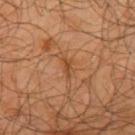<record>
  <biopsy_status>not biopsied; imaged during a skin examination</biopsy_status>
  <image>
    <source>total-body photography crop</source>
    <field_of_view_mm>15</field_of_view_mm>
  </image>
  <automated_metrics>
    <vs_skin_darker_L>8.0</vs_skin_darker_L>
    <nevus_likeness_0_100>0</nevus_likeness_0_100>
    <lesion_detection_confidence_0_100>95</lesion_detection_confidence_0_100>
  </automated_metrics>
  <lesion_size>
    <long_diameter_mm_approx>2.5</long_diameter_mm_approx>
  </lesion_size>
  <site>arm</site>
  <patient>
    <sex>male</sex>
    <age_approx>65</age_approx>
  </patient>
</record>